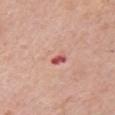| field | value |
|---|---|
| follow-up | imaged on a skin check; not biopsied |
| image source | 15 mm crop, total-body photography |
| site | the arm |
| diameter | about 3 mm |
| patient | female, in their mid- to late 60s |